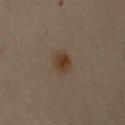Case summary:
* biopsy status — no biopsy performed (imaged during a skin exam)
* image — 15 mm crop, total-body photography
* patient — female, in their mid-40s
* body site — the right upper arm
* tile lighting — cross-polarized
* image-analysis metrics — a footprint of about 5 mm² and a shape eccentricity near 0.5; a lesion color around L≈31 a*≈12 b*≈23 in CIELAB, about 7 CIELAB-L* units darker than the surrounding skin, and a normalized lesion–skin contrast near 8.5; a classifier nevus-likeness of about 95/100 and a detector confidence of about 100 out of 100 that the crop contains a lesion
* lesion diameter — ≈3 mm